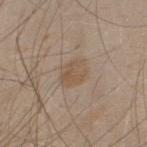Impression:
This lesion was catalogued during total-body skin photography and was not selected for biopsy.
Acquisition and patient details:
A male patient, aged approximately 30. A region of skin cropped from a whole-body photographic capture, roughly 15 mm wide. The lesion is located on the upper back.